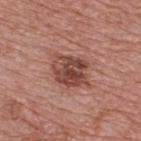<case>
  <biopsy_status>not biopsied; imaged during a skin examination</biopsy_status>
  <lesion_size>
    <long_diameter_mm_approx>4.5</long_diameter_mm_approx>
  </lesion_size>
  <site>upper back</site>
  <lighting>white-light</lighting>
  <automated_metrics>
    <area_mm2_approx>12.0</area_mm2_approx>
    <eccentricity>0.6</eccentricity>
    <shape_asymmetry>0.25</shape_asymmetry>
    <cielab_L>45</cielab_L>
    <cielab_a>24</cielab_a>
    <cielab_b>25</cielab_b>
    <vs_skin_darker_L>12.0</vs_skin_darker_L>
    <vs_skin_contrast_norm>9.0</vs_skin_contrast_norm>
    <border_irregularity_0_10>3.0</border_irregularity_0_10>
    <color_variation_0_10>6.5</color_variation_0_10>
    <peripheral_color_asymmetry>2.5</peripheral_color_asymmetry>
    <lesion_detection_confidence_0_100>100</lesion_detection_confidence_0_100>
  </automated_metrics>
  <image>
    <source>total-body photography crop</source>
    <field_of_view_mm>15</field_of_view_mm>
  </image>
  <patient>
    <sex>male</sex>
    <age_approx>70</age_approx>
  </patient>
</case>